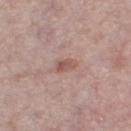Impression:
No biopsy was performed on this lesion — it was imaged during a full skin examination and was not determined to be concerning.
Acquisition and patient details:
This is a white-light tile. A region of skin cropped from a whole-body photographic capture, roughly 15 mm wide. Approximately 3 mm at its widest. Automated image analysis of the tile measured roughly 9 lightness units darker than nearby skin and a lesion-to-skin contrast of about 6.5 (normalized; higher = more distinct). The software also gave a classifier nevus-likeness of about 0/100 and lesion-presence confidence of about 100/100. A female subject aged 58–62. On the right thigh.